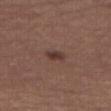| key | value |
|---|---|
| workup | catalogued during a skin exam; not biopsied |
| lesion size | ~2.5 mm (longest diameter) |
| acquisition | 15 mm crop, total-body photography |
| body site | the front of the torso |
| subject | male, aged 68 to 72 |
| illumination | white-light illumination |